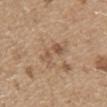Part of a total-body skin-imaging series; this lesion was reviewed on a skin check and was not flagged for biopsy. The lesion is on the left upper arm. This is a white-light tile. A male subject, aged 48 to 52. Cropped from a whole-body photographic skin survey; the tile spans about 15 mm.